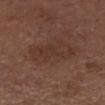Findings:
* patient: male, approximately 75 years of age
* image source: ~15 mm tile from a whole-body skin photo
* body site: the head or neck
* size: ≈6.5 mm